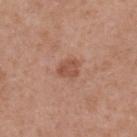Clinical impression:
Captured during whole-body skin photography for melanoma surveillance; the lesion was not biopsied.
Image and clinical context:
A female subject, aged 38–42. On the upper back. About 2.5 mm across. This is a white-light tile. A 15 mm close-up tile from a total-body photography series done for melanoma screening.